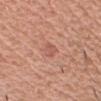  biopsy_status: not biopsied; imaged during a skin examination
  site: head or neck
  lighting: white-light
  patient:
    sex: male
    age_approx: 60
  image:
    source: total-body photography crop
    field_of_view_mm: 15